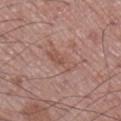Notes:
* TBP lesion metrics — a lesion area of about 5 mm² and an outline eccentricity of about 0.9 (0 = round, 1 = elongated); a lesion color around L≈52 a*≈21 b*≈26 in CIELAB, about 7 CIELAB-L* units darker than the surrounding skin, and a normalized border contrast of about 5.5
* location — the right lower leg
* image source — total-body-photography crop, ~15 mm field of view
* subject — male, aged approximately 70
* lesion diameter — ≈4 mm
* lighting — white-light